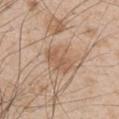Impression: Part of a total-body skin-imaging series; this lesion was reviewed on a skin check and was not flagged for biopsy. Background: A 15 mm close-up tile from a total-body photography series done for melanoma screening. The lesion's longest dimension is about 4 mm. A male subject about 50 years old. The tile uses white-light illumination. From the left upper arm.